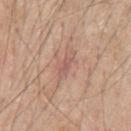biopsy status: catalogued during a skin exam; not biopsied | patient: male, aged 58 to 62 | image: ~15 mm crop, total-body skin-cancer survey | anatomic site: the right upper arm | illumination: white-light illumination | lesion size: ≈4 mm.